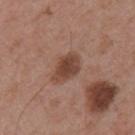• patient: male, aged 53–57
• image-analysis metrics: a footprint of about 7.5 mm², an eccentricity of roughly 0.75, and two-axis asymmetry of about 0.2; about 11 CIELAB-L* units darker than the surrounding skin and a normalized border contrast of about 9; internal color variation of about 3 on a 0–10 scale; a classifier nevus-likeness of about 70/100
• location: the mid back
• size: ~4 mm (longest diameter)
• image source: ~15 mm tile from a whole-body skin photo
• illumination: white-light illumination